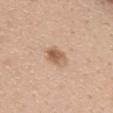Captured during whole-body skin photography for melanoma surveillance; the lesion was not biopsied. On the upper back. The lesion's longest dimension is about 3 mm. Cropped from a total-body skin-imaging series; the visible field is about 15 mm. A female subject, approximately 25 years of age.